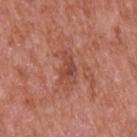Notes:
- workup: imaged on a skin check; not biopsied
- TBP lesion metrics: a footprint of about 5 mm², a shape eccentricity near 0.8, and a symmetry-axis asymmetry near 0.45; a lesion color around L≈47 a*≈27 b*≈29 in CIELAB and a normalized lesion–skin contrast near 6
- patient: male, aged 63 to 67
- tile lighting: white-light illumination
- image: ~15 mm tile from a whole-body skin photo
- site: the upper back